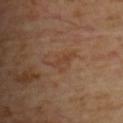follow-up: imaged on a skin check; not biopsied
acquisition: ~15 mm crop, total-body skin-cancer survey
automated lesion analysis: a footprint of about 2 mm², an outline eccentricity of about 0.95 (0 = round, 1 = elongated), and a symmetry-axis asymmetry near 0.3; a lesion–skin lightness drop of about 5; a classifier nevus-likeness of about 0/100 and a lesion-detection confidence of about 100/100
body site: the front of the torso
illumination: cross-polarized
patient: female